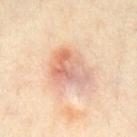Acquisition and patient details:
Approximately 5 mm at its widest. Cropped from a whole-body photographic skin survey; the tile spans about 15 mm. The lesion is located on the chest. The total-body-photography lesion software estimated a footprint of about 12 mm², an outline eccentricity of about 0.7 (0 = round, 1 = elongated), and a shape-asymmetry score of about 0.45 (0 = symmetric). The software also gave a border-irregularity index near 5/10, a within-lesion color-variation index near 7/10, and peripheral color asymmetry of about 2. The software also gave an automated nevus-likeness rating near 0 out of 100 and a detector confidence of about 100 out of 100 that the crop contains a lesion. Imaged with cross-polarized lighting. The subject is a male in their mid- to late 30s.